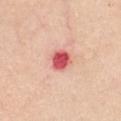The lesion was photographed on a routine skin check and not biopsied; there is no pathology result. A region of skin cropped from a whole-body photographic capture, roughly 15 mm wide. A female patient, aged around 55. The tile uses white-light illumination. The lesion-visualizer software estimated an eccentricity of roughly 0.5 and a symmetry-axis asymmetry near 0.15. The lesion's longest dimension is about 2.5 mm. The lesion is on the chest.